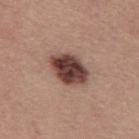{
  "biopsy_status": "not biopsied; imaged during a skin examination",
  "lesion_size": {
    "long_diameter_mm_approx": 4.5
  },
  "site": "mid back",
  "image": {
    "source": "total-body photography crop",
    "field_of_view_mm": 15
  },
  "patient": {
    "sex": "male",
    "age_approx": 40
  }
}